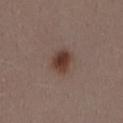biopsy status: total-body-photography surveillance lesion; no biopsy | site: the mid back | image source: ~15 mm crop, total-body skin-cancer survey | patient: female, aged around 30.